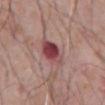The lesion was photographed on a routine skin check and not biopsied; there is no pathology result.
Approximately 4 mm at its widest.
A 15 mm crop from a total-body photograph taken for skin-cancer surveillance.
The patient is a male aged 58 to 62.
The tile uses white-light illumination.
The lesion is located on the abdomen.